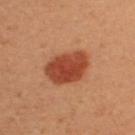| feature | finding |
|---|---|
| notes | no biopsy performed (imaged during a skin exam) |
| body site | the left upper arm |
| lesion size | ≈5.5 mm |
| patient | female, aged around 30 |
| illumination | cross-polarized |
| acquisition | total-body-photography crop, ~15 mm field of view |
| TBP lesion metrics | border irregularity of about 2.5 on a 0–10 scale, a within-lesion color-variation index near 3.5/10, and radial color variation of about 1 |